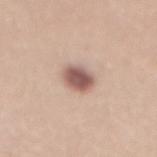No biopsy was performed on this lesion — it was imaged during a full skin examination and was not determined to be concerning.
Located on the back.
The subject is a female about 35 years old.
Approximately 3.5 mm at its widest.
This is a white-light tile.
A 15 mm close-up tile from a total-body photography series done for melanoma screening.